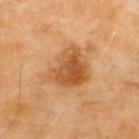{
  "biopsy_status": "not biopsied; imaged during a skin examination",
  "automated_metrics": {
    "area_mm2_approx": 16.0,
    "eccentricity": 0.55,
    "shape_asymmetry": 0.3
  },
  "site": "back",
  "image": {
    "source": "total-body photography crop",
    "field_of_view_mm": 15
  },
  "patient": {
    "sex": "male",
    "age_approx": 70
  }
}